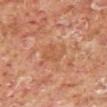Q: Was this lesion biopsied?
A: imaged on a skin check; not biopsied
Q: How was the tile lit?
A: cross-polarized
Q: What is the lesion's diameter?
A: about 3.5 mm
Q: Who is the patient?
A: male, about 60 years old
Q: What is the imaging modality?
A: ~15 mm tile from a whole-body skin photo
Q: Where on the body is the lesion?
A: the left lower leg